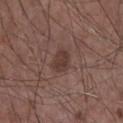Clinical impression:
No biopsy was performed on this lesion — it was imaged during a full skin examination and was not determined to be concerning.
Context:
The total-body-photography lesion software estimated an eccentricity of roughly 0.7 and two-axis asymmetry of about 0.2. The software also gave a lesion-detection confidence of about 100/100. On the chest. About 3 mm across. This image is a 15 mm lesion crop taken from a total-body photograph. A male patient, approximately 60 years of age. Captured under white-light illumination.